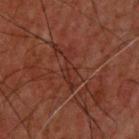notes: catalogued during a skin exam; not biopsied
location: the upper back
imaging modality: ~15 mm tile from a whole-body skin photo
tile lighting: cross-polarized illumination
TBP lesion metrics: an outline eccentricity of about 0.95 (0 = round, 1 = elongated) and two-axis asymmetry of about 0.55; a nevus-likeness score of about 0/100 and lesion-presence confidence of about 50/100
diameter: ~5.5 mm (longest diameter)
subject: male, aged 58–62